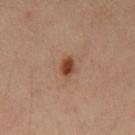Imaged during a routine full-body skin examination; the lesion was not biopsied and no histopathology is available.
The recorded lesion diameter is about 2.5 mm.
This is a cross-polarized tile.
A 15 mm close-up tile from a total-body photography series done for melanoma screening.
A male patient, about 60 years old.
The lesion is located on the chest.
An algorithmic analysis of the crop reported a footprint of about 3.5 mm², an eccentricity of roughly 0.8, and a shape-asymmetry score of about 0.25 (0 = symmetric). And it measured an automated nevus-likeness rating near 100 out of 100 and a detector confidence of about 100 out of 100 that the crop contains a lesion.